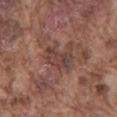Acquisition and patient details:
Measured at roughly 4 mm in maximum diameter. The lesion is located on the mid back. A male patient aged 73 to 77. Captured under white-light illumination. A 15 mm close-up tile from a total-body photography series done for melanoma screening.